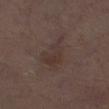notes = catalogued during a skin exam; not biopsied
tile lighting = white-light
acquisition = 15 mm crop, total-body photography
lesion diameter = about 5.5 mm
body site = the right thigh
subject = male, aged 68–72